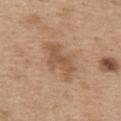{
  "biopsy_status": "not biopsied; imaged during a skin examination",
  "site": "upper back",
  "image": {
    "source": "total-body photography crop",
    "field_of_view_mm": 15
  },
  "lesion_size": {
    "long_diameter_mm_approx": 5.5
  },
  "patient": {
    "sex": "female",
    "age_approx": 45
  }
}